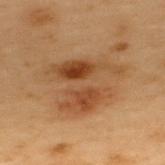The lesion was tiled from a total-body skin photograph and was not biopsied. Longest diameter approximately 7.5 mm. A male patient roughly 55 years of age. A region of skin cropped from a whole-body photographic capture, roughly 15 mm wide. Captured under cross-polarized illumination. The lesion is located on the upper back. Automated tile analysis of the lesion measured an area of roughly 23 mm², an eccentricity of roughly 0.45, and two-axis asymmetry of about 0.45. The software also gave an average lesion color of about L≈38 a*≈18 b*≈31 (CIELAB), a lesion–skin lightness drop of about 9, and a normalized lesion–skin contrast near 8. The software also gave border irregularity of about 7 on a 0–10 scale, a within-lesion color-variation index near 7.5/10, and radial color variation of about 2.5.